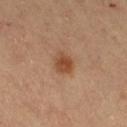<tbp_lesion>
  <biopsy_status>not biopsied; imaged during a skin examination</biopsy_status>
  <patient>
    <sex>female</sex>
    <age_approx>60</age_approx>
  </patient>
  <automated_metrics>
    <area_mm2_approx>5.0</area_mm2_approx>
    <eccentricity>0.45</eccentricity>
    <shape_asymmetry>0.2</shape_asymmetry>
    <vs_skin_darker_L>11.0</vs_skin_darker_L>
    <vs_skin_contrast_norm>8.0</vs_skin_contrast_norm>
    <border_irregularity_0_10>1.5</border_irregularity_0_10>
    <color_variation_0_10>2.5</color_variation_0_10>
    <peripheral_color_asymmetry>1.0</peripheral_color_asymmetry>
    <nevus_likeness_0_100>95</nevus_likeness_0_100>
    <lesion_detection_confidence_0_100>100</lesion_detection_confidence_0_100>
  </automated_metrics>
  <image>
    <source>total-body photography crop</source>
    <field_of_view_mm>15</field_of_view_mm>
  </image>
  <site>right thigh</site>
  <lighting>cross-polarized</lighting>
</tbp_lesion>